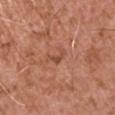Impression:
This lesion was catalogued during total-body skin photography and was not selected for biopsy.
Acquisition and patient details:
A region of skin cropped from a whole-body photographic capture, roughly 15 mm wide. A male subject, roughly 75 years of age. From the left upper arm. The total-body-photography lesion software estimated a shape eccentricity near 0.85 and a shape-asymmetry score of about 0.6 (0 = symmetric). And it measured a classifier nevus-likeness of about 0/100 and lesion-presence confidence of about 100/100. This is a white-light tile.